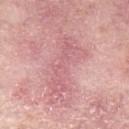workup — no biopsy performed (imaged during a skin exam)
automated lesion analysis — border irregularity of about 7.5 on a 0–10 scale, a within-lesion color-variation index near 2.5/10, and a peripheral color-asymmetry measure near 0.5; an automated nevus-likeness rating near 0 out of 100 and lesion-presence confidence of about 55/100
illumination — white-light illumination
body site — the left upper arm
image — ~15 mm crop, total-body skin-cancer survey
patient — female, aged 68–72